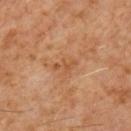Part of a total-body skin-imaging series; this lesion was reviewed on a skin check and was not flagged for biopsy.
The tile uses cross-polarized illumination.
A region of skin cropped from a whole-body photographic capture, roughly 15 mm wide.
Automated tile analysis of the lesion measured a mean CIELAB color near L≈49 a*≈22 b*≈35, about 6 CIELAB-L* units darker than the surrounding skin, and a lesion-to-skin contrast of about 5.5 (normalized; higher = more distinct). It also reported a nevus-likeness score of about 0/100 and a lesion-detection confidence of about 100/100.
From the chest.
A male subject about 60 years old.
About 3 mm across.